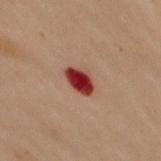Assessment: Recorded during total-body skin imaging; not selected for excision or biopsy. Clinical summary: The total-body-photography lesion software estimated a footprint of about 6.5 mm² and an eccentricity of roughly 0.7. The subject is a female aged 58–62. The tile uses cross-polarized illumination. The lesion is on the upper back. Cropped from a whole-body photographic skin survey; the tile spans about 15 mm. The lesion's longest dimension is about 3.5 mm.